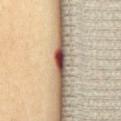The lesion was tiled from a total-body skin photograph and was not biopsied.
This is a cross-polarized tile.
On the chest.
A female subject, aged approximately 55.
The total-body-photography lesion software estimated an area of roughly 3 mm² and an outline eccentricity of about 0.9 (0 = round, 1 = elongated). The software also gave a mean CIELAB color near L≈44 a*≈27 b*≈25, roughly 27 lightness units darker than nearby skin, and a normalized lesion–skin contrast near 18.
Cropped from a total-body skin-imaging series; the visible field is about 15 mm.
The lesion's longest dimension is about 3 mm.